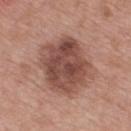image-analysis metrics=two-axis asymmetry of about 0.15; internal color variation of about 6.5 on a 0–10 scale; a nevus-likeness score of about 20/100 and a lesion-detection confidence of about 100/100 | location=the upper back | image source=total-body-photography crop, ~15 mm field of view | subject=male, in their mid-60s | illumination=white-light illumination | lesion diameter=≈6.5 mm.